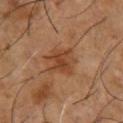Imaged during a routine full-body skin examination; the lesion was not biopsied and no histopathology is available. A male patient aged 53 to 57. The lesion-visualizer software estimated a footprint of about 8.5 mm². And it measured a lesion color around L≈42 a*≈22 b*≈33 in CIELAB and a normalized border contrast of about 7. The software also gave a lesion-detection confidence of about 100/100. A 15 mm crop from a total-body photograph taken for skin-cancer surveillance. On the front of the torso. Measured at roughly 3.5 mm in maximum diameter.